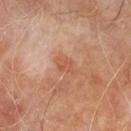<record>
  <biopsy_status>not biopsied; imaged during a skin examination</biopsy_status>
  <lesion_size>
    <long_diameter_mm_approx>2.5</long_diameter_mm_approx>
  </lesion_size>
  <automated_metrics>
    <area_mm2_approx>3.0</area_mm2_approx>
    <eccentricity>0.85</eccentricity>
    <shape_asymmetry>0.5</shape_asymmetry>
    <cielab_L>52</cielab_L>
    <cielab_a>24</cielab_a>
    <cielab_b>32</cielab_b>
    <vs_skin_darker_L>6.0</vs_skin_darker_L>
    <vs_skin_contrast_norm>5.0</vs_skin_contrast_norm>
  </automated_metrics>
  <site>leg</site>
  <image>
    <source>total-body photography crop</source>
    <field_of_view_mm>15</field_of_view_mm>
  </image>
  <patient>
    <sex>male</sex>
    <age_approx>60</age_approx>
  </patient>
  <lighting>cross-polarized</lighting>
</record>